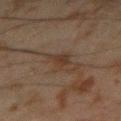<tbp_lesion>
<biopsy_status>not biopsied; imaged during a skin examination</biopsy_status>
<automated_metrics>
  <area_mm2_approx>4.0</area_mm2_approx>
  <eccentricity>0.85</eccentricity>
  <shape_asymmetry>0.4</shape_asymmetry>
  <cielab_L>28</cielab_L>
  <cielab_a>12</cielab_a>
  <cielab_b>21</cielab_b>
  <vs_skin_darker_L>6.0</vs_skin_darker_L>
  <vs_skin_contrast_norm>7.0</vs_skin_contrast_norm>
  <nevus_likeness_0_100>20</nevus_likeness_0_100>
  <lesion_detection_confidence_0_100>100</lesion_detection_confidence_0_100>
</automated_metrics>
<lesion_size>
  <long_diameter_mm_approx>3.0</long_diameter_mm_approx>
</lesion_size>
<patient>
  <sex>male</sex>
  <age_approx>45</age_approx>
</patient>
<lighting>cross-polarized</lighting>
<site>right forearm</site>
<image>
  <source>total-body photography crop</source>
  <field_of_view_mm>15</field_of_view_mm>
</image>
</tbp_lesion>